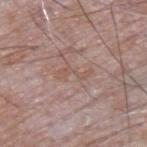<tbp_lesion>
  <biopsy_status>not biopsied; imaged during a skin examination</biopsy_status>
  <site>upper back</site>
  <automated_metrics>
    <area_mm2_approx>4.0</area_mm2_approx>
    <vs_skin_darker_L>5.0</vs_skin_darker_L>
    <vs_skin_contrast_norm>5.0</vs_skin_contrast_norm>
    <nevus_likeness_0_100>0</nevus_likeness_0_100>
  </automated_metrics>
  <patient>
    <sex>male</sex>
    <age_approx>65</age_approx>
  </patient>
  <lighting>white-light</lighting>
  <image>
    <source>total-body photography crop</source>
    <field_of_view_mm>15</field_of_view_mm>
  </image>
</tbp_lesion>